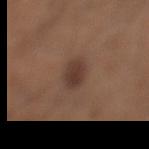Case summary:
– notes · imaged on a skin check; not biopsied
– subject · male, roughly 30 years of age
– site · the leg
– lesion diameter · ~3 mm (longest diameter)
– acquisition · total-body-photography crop, ~15 mm field of view
– lighting · white-light